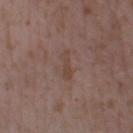This lesion was catalogued during total-body skin photography and was not selected for biopsy.
An algorithmic analysis of the crop reported an area of roughly 3.5 mm² and two-axis asymmetry of about 0.35. And it measured border irregularity of about 4.5 on a 0–10 scale and peripheral color asymmetry of about 0.
The patient is a female about 35 years old.
Approximately 3.5 mm at its widest.
The lesion is located on the right forearm.
Captured under white-light illumination.
Cropped from a whole-body photographic skin survey; the tile spans about 15 mm.